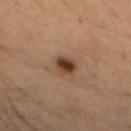notes = imaged on a skin check; not biopsied
subject = male, aged 48–52
TBP lesion metrics = a border-irregularity index near 2/10, a within-lesion color-variation index near 5.5/10, and radial color variation of about 1.5
tile lighting = cross-polarized illumination
image = ~15 mm crop, total-body skin-cancer survey
body site = the mid back
lesion size = ~2.5 mm (longest diameter)